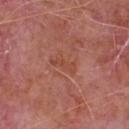{
  "biopsy_status": "not biopsied; imaged during a skin examination",
  "lesion_size": {
    "long_diameter_mm_approx": 3.0
  },
  "patient": {
    "sex": "male",
    "age_approx": 65
  },
  "site": "chest",
  "image": {
    "source": "total-body photography crop",
    "field_of_view_mm": 15
  },
  "lighting": "white-light"
}